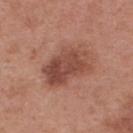Clinical impression:
Captured during whole-body skin photography for melanoma surveillance; the lesion was not biopsied.
Clinical summary:
The patient is a female roughly 40 years of age. Captured under white-light illumination. The lesion-visualizer software estimated a lesion–skin lightness drop of about 11 and a normalized border contrast of about 8. The analysis additionally found an automated nevus-likeness rating near 25 out of 100. On the upper back. A roughly 15 mm field-of-view crop from a total-body skin photograph.